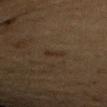Case summary:
• follow-up · imaged on a skin check; not biopsied
• image source · total-body-photography crop, ~15 mm field of view
• site · the right upper arm
• diameter · ≈2.5 mm
• illumination · cross-polarized illumination
• subject · female, roughly 40 years of age